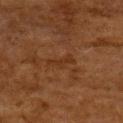notes — imaged on a skin check; not biopsied | image-analysis metrics — an average lesion color of about L≈26 a*≈18 b*≈28 (CIELAB) and roughly 4 lightness units darker than nearby skin; a within-lesion color-variation index near 0/10 and radial color variation of about 0; a classifier nevus-likeness of about 0/100 and lesion-presence confidence of about 100/100 | acquisition — ~15 mm crop, total-body skin-cancer survey | lesion size — ~3.5 mm (longest diameter) | subject — female, about 50 years old | illumination — cross-polarized illumination | site — the upper back.